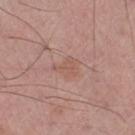biopsy_status: not biopsied; imaged during a skin examination
site: right lower leg
patient:
  sex: male
  age_approx: 70
image:
  source: total-body photography crop
  field_of_view_mm: 15
lighting: white-light
lesion_size:
  long_diameter_mm_approx: 3.0